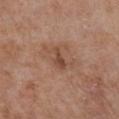<case>
<biopsy_status>not biopsied; imaged during a skin examination</biopsy_status>
<site>front of the torso</site>
<automated_metrics>
  <area_mm2_approx>3.0</area_mm2_approx>
  <eccentricity>0.9</eccentricity>
  <shape_asymmetry>0.5</shape_asymmetry>
  <border_irregularity_0_10>5.0</border_irregularity_0_10>
  <color_variation_0_10>1.0</color_variation_0_10>
  <peripheral_color_asymmetry>0.0</peripheral_color_asymmetry>
  <nevus_likeness_0_100>0</nevus_likeness_0_100>
</automated_metrics>
<patient>
  <sex>female</sex>
  <age_approx>80</age_approx>
</patient>
<lesion_size>
  <long_diameter_mm_approx>3.0</long_diameter_mm_approx>
</lesion_size>
<image>
  <source>total-body photography crop</source>
  <field_of_view_mm>15</field_of_view_mm>
</image>
<lighting>white-light</lighting>
</case>